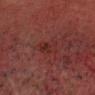  biopsy_status: not biopsied; imaged during a skin examination
  site: head or neck
  automated_metrics:
    eccentricity: 0.85
    shape_asymmetry: 0.25
    lesion_detection_confidence_0_100: 100
  lesion_size:
    long_diameter_mm_approx: 3.5
  image:
    source: total-body photography crop
    field_of_view_mm: 15
  lighting: cross-polarized
  patient:
    sex: male
    age_approx: 50